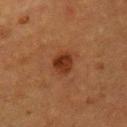Case summary:
- notes · catalogued during a skin exam; not biopsied
- image source · 15 mm crop, total-body photography
- tile lighting · cross-polarized
- location · the chest
- size · ~3 mm (longest diameter)
- patient · male, in their mid- to late 70s